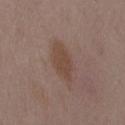biopsy status: total-body-photography surveillance lesion; no biopsy
size: about 4.5 mm
anatomic site: the mid back
automated metrics: a footprint of about 7.5 mm², an eccentricity of roughly 0.85, and a shape-asymmetry score of about 0.25 (0 = symmetric); a border-irregularity rating of about 3/10 and a color-variation rating of about 2/10
lighting: white-light illumination
patient: female, in their mid- to late 30s
acquisition: 15 mm crop, total-body photography